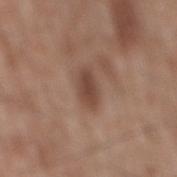The recorded lesion diameter is about 3.5 mm.
The lesion-visualizer software estimated a lesion color around L≈44 a*≈19 b*≈26 in CIELAB, roughly 11 lightness units darker than nearby skin, and a normalized border contrast of about 8. The analysis additionally found a border-irregularity index near 2/10, a color-variation rating of about 2/10, and peripheral color asymmetry of about 0.5.
A male subject, in their 60s.
The lesion is on the back.
This is a white-light tile.
A lesion tile, about 15 mm wide, cut from a 3D total-body photograph.